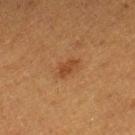Notes:
- biopsy status · no biopsy performed (imaged during a skin exam)
- TBP lesion metrics · a border-irregularity index near 2.5/10, a within-lesion color-variation index near 1/10, and peripheral color asymmetry of about 0.5
- anatomic site · the right lower leg
- lesion diameter · ≈2.5 mm
- tile lighting · cross-polarized illumination
- image · ~15 mm tile from a whole-body skin photo
- patient · female, aged 38–42